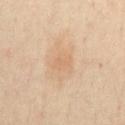notes = total-body-photography surveillance lesion; no biopsy | tile lighting = cross-polarized illumination | diameter = about 2.5 mm | patient = male, about 50 years old | location = the front of the torso | acquisition = 15 mm crop, total-body photography.